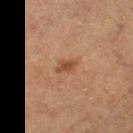{
  "automated_metrics": {
    "cielab_L": 38,
    "cielab_a": 19,
    "cielab_b": 29,
    "vs_skin_darker_L": 8.0,
    "vs_skin_contrast_norm": 7.5,
    "border_irregularity_0_10": 3.5,
    "color_variation_0_10": 0.5,
    "nevus_likeness_0_100": 65
  },
  "lesion_size": {
    "long_diameter_mm_approx": 2.5
  },
  "patient": {
    "sex": "male",
    "age_approx": 75
  },
  "lighting": "cross-polarized",
  "site": "right thigh",
  "image": {
    "source": "total-body photography crop",
    "field_of_view_mm": 15
  }
}